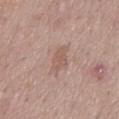This lesion was catalogued during total-body skin photography and was not selected for biopsy. The subject is a male in their 70s. Located on the mid back. A 15 mm crop from a total-body photograph taken for skin-cancer surveillance.